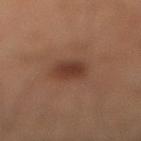follow-up = no biopsy performed (imaged during a skin exam)
lighting = cross-polarized
body site = the leg
image source = ~15 mm tile from a whole-body skin photo
subject = male, roughly 60 years of age
lesion size = about 3 mm